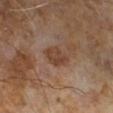| feature | finding |
|---|---|
| biopsy status | catalogued during a skin exam; not biopsied |
| patient | male, aged approximately 65 |
| image-analysis metrics | an average lesion color of about L≈40 a*≈19 b*≈27 (CIELAB), roughly 8 lightness units darker than nearby skin, and a normalized border contrast of about 7.5 |
| tile lighting | cross-polarized illumination |
| imaging modality | 15 mm crop, total-body photography |